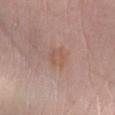notes — no biopsy performed (imaged during a skin exam) | patient — female, roughly 65 years of age | diameter — about 2.5 mm | location — the left lower leg | image — ~15 mm tile from a whole-body skin photo | automated metrics — an area of roughly 4.5 mm², an outline eccentricity of about 0.55 (0 = round, 1 = elongated), and a symmetry-axis asymmetry near 0.2; an average lesion color of about L≈55 a*≈19 b*≈26 (CIELAB), a lesion–skin lightness drop of about 6, and a normalized border contrast of about 5; border irregularity of about 2 on a 0–10 scale and a peripheral color-asymmetry measure near 1 | illumination — white-light illumination.